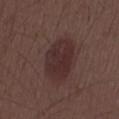Q: Was a biopsy performed?
A: imaged on a skin check; not biopsied